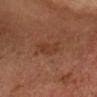Case summary:
• follow-up — catalogued during a skin exam; not biopsied
• acquisition — ~15 mm tile from a whole-body skin photo
• lesion size — ~3.5 mm (longest diameter)
• site — the head or neck
• lighting — cross-polarized
• patient — male, in their mid-60s